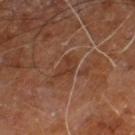Notes:
- follow-up — imaged on a skin check; not biopsied
- image — ~15 mm crop, total-body skin-cancer survey
- body site — the right leg
- lighting — cross-polarized
- subject — male, about 60 years old
- size — ≈3 mm